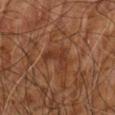Impression:
Imaged during a routine full-body skin examination; the lesion was not biopsied and no histopathology is available.